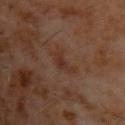Captured during whole-body skin photography for melanoma surveillance; the lesion was not biopsied. The patient is a male about 60 years old. A 15 mm crop from a total-body photograph taken for skin-cancer surveillance. Located on the back.